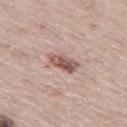lesion size: ~3.5 mm (longest diameter)
image: ~15 mm crop, total-body skin-cancer survey
illumination: white-light illumination
body site: the left thigh
image-analysis metrics: an area of roughly 6 mm², an outline eccentricity of about 0.85 (0 = round, 1 = elongated), and two-axis asymmetry of about 0.2; a nevus-likeness score of about 65/100 and lesion-presence confidence of about 100/100
subject: male, in their mid- to late 60s